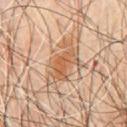Imaged during a routine full-body skin examination; the lesion was not biopsied and no histopathology is available. From the front of the torso. This image is a 15 mm lesion crop taken from a total-body photograph. A male patient roughly 70 years of age.